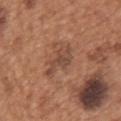Clinical summary:
A male patient in their mid-60s. This image is a 15 mm lesion crop taken from a total-body photograph. Approximately 5 mm at its widest. On the upper back. Automated image analysis of the tile measured a lesion area of about 6.5 mm², an outline eccentricity of about 0.9 (0 = round, 1 = elongated), and a shape-asymmetry score of about 0.5 (0 = symmetric). The analysis additionally found about 8 CIELAB-L* units darker than the surrounding skin. And it measured border irregularity of about 6.5 on a 0–10 scale, a within-lesion color-variation index near 3/10, and peripheral color asymmetry of about 1. And it measured a nevus-likeness score of about 0/100.